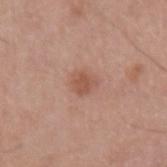Recorded during total-body skin imaging; not selected for excision or biopsy. A male patient, in their mid-60s. From the left upper arm. A roughly 15 mm field-of-view crop from a total-body skin photograph. The lesion's longest dimension is about 2.5 mm.